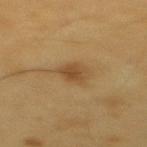Recorded during total-body skin imaging; not selected for excision or biopsy.
Automated image analysis of the tile measured an eccentricity of roughly 0.6 and a symmetry-axis asymmetry near 0.25. And it measured an average lesion color of about L≈47 a*≈17 b*≈37 (CIELAB), roughly 9 lightness units darker than nearby skin, and a lesion-to-skin contrast of about 7 (normalized; higher = more distinct). It also reported internal color variation of about 2 on a 0–10 scale and a peripheral color-asymmetry measure near 1. The analysis additionally found a classifier nevus-likeness of about 90/100 and lesion-presence confidence of about 100/100.
Longest diameter approximately 3 mm.
The tile uses cross-polarized illumination.
Located on the upper back.
This image is a 15 mm lesion crop taken from a total-body photograph.
The subject is a male roughly 60 years of age.